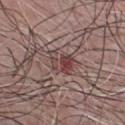* subject: male, aged 63 to 67
* body site: the chest
* acquisition: ~15 mm crop, total-body skin-cancer survey